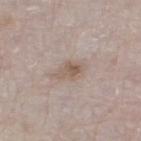Imaged during a routine full-body skin examination; the lesion was not biopsied and no histopathology is available. The subject is a male roughly 80 years of age. A 15 mm close-up tile from a total-body photography series done for melanoma screening. From the leg.